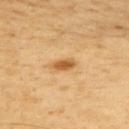| feature | finding |
|---|---|
| acquisition | ~15 mm tile from a whole-body skin photo |
| illumination | cross-polarized |
| anatomic site | the upper back |
| automated lesion analysis | a border-irregularity index near 1.5/10, internal color variation of about 2 on a 0–10 scale, and radial color variation of about 0.5; a nevus-likeness score of about 100/100 and a lesion-detection confidence of about 100/100 |
| lesion diameter | ≈3 mm |
| patient | male, about 60 years old |